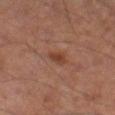Context:
A 15 mm close-up tile from a total-body photography series done for melanoma screening. A male subject, about 55 years old. The lesion-visualizer software estimated a color-variation rating of about 1.5/10 and radial color variation of about 0.5. From the left thigh. The lesion's longest dimension is about 2.5 mm. Captured under cross-polarized illumination.